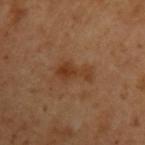Findings:
– biopsy status: no biopsy performed (imaged during a skin exam)
– patient: male, approximately 50 years of age
– body site: the left upper arm
– image: ~15 mm tile from a whole-body skin photo